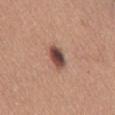Part of a total-body skin-imaging series; this lesion was reviewed on a skin check and was not flagged for biopsy. Approximately 3.5 mm at its widest. Imaged with white-light lighting. The lesion is on the chest. The patient is a female aged around 50. A close-up tile cropped from a whole-body skin photograph, about 15 mm across.